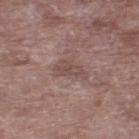Clinical impression: Imaged during a routine full-body skin examination; the lesion was not biopsied and no histopathology is available. Image and clinical context: A male subject in their 70s. Cropped from a whole-body photographic skin survey; the tile spans about 15 mm. From the right lower leg. The recorded lesion diameter is about 3.5 mm. Automated tile analysis of the lesion measured a shape-asymmetry score of about 0.35 (0 = symmetric). And it measured a mean CIELAB color near L≈48 a*≈18 b*≈21, a lesion–skin lightness drop of about 7, and a lesion-to-skin contrast of about 5.5 (normalized; higher = more distinct).